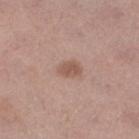Q: Was a biopsy performed?
A: no biopsy performed (imaged during a skin exam)
Q: What is the imaging modality?
A: total-body-photography crop, ~15 mm field of view
Q: What is the lesion's diameter?
A: ~2.5 mm (longest diameter)
Q: How was the tile lit?
A: white-light illumination
Q: Lesion location?
A: the right lower leg
Q: What did automated image analysis measure?
A: an area of roughly 4 mm², an outline eccentricity of about 0.7 (0 = round, 1 = elongated), and a shape-asymmetry score of about 0.2 (0 = symmetric); a lesion color around L≈54 a*≈19 b*≈26 in CIELAB, a lesion–skin lightness drop of about 9, and a normalized lesion–skin contrast near 7; a border-irregularity index near 2/10 and a within-lesion color-variation index near 1.5/10; a nevus-likeness score of about 60/100 and a detector confidence of about 100 out of 100 that the crop contains a lesion
Q: What are the patient's age and sex?
A: female, roughly 40 years of age